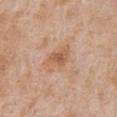Impression: Imaged during a routine full-body skin examination; the lesion was not biopsied and no histopathology is available. Background: Automated image analysis of the tile measured a border-irregularity index near 3.5/10 and internal color variation of about 3.5 on a 0–10 scale. And it measured lesion-presence confidence of about 100/100. Longest diameter approximately 4 mm. A male subject roughly 65 years of age. On the front of the torso. A close-up tile cropped from a whole-body skin photograph, about 15 mm across. This is a white-light tile.